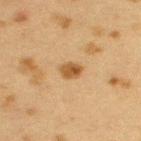Assessment:
No biopsy was performed on this lesion — it was imaged during a full skin examination and was not determined to be concerning.
Acquisition and patient details:
A female subject aged 38–42. A 15 mm close-up extracted from a 3D total-body photography capture. The lesion is on the left upper arm. An algorithmic analysis of the crop reported a mean CIELAB color near L≈45 a*≈17 b*≈35, about 10 CIELAB-L* units darker than the surrounding skin, and a normalized lesion–skin contrast near 8.5. The analysis additionally found a border-irregularity index near 2/10 and peripheral color asymmetry of about 1.5. It also reported an automated nevus-likeness rating near 95 out of 100 and a detector confidence of about 100 out of 100 that the crop contains a lesion. This is a cross-polarized tile.